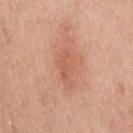Clinical impression:
This lesion was catalogued during total-body skin photography and was not selected for biopsy.
Clinical summary:
A 15 mm close-up tile from a total-body photography series done for melanoma screening. The lesion is located on the upper back. The subject is a male aged around 45. The recorded lesion diameter is about 4 mm. The tile uses white-light illumination.